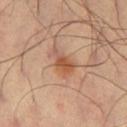  biopsy_status: not biopsied; imaged during a skin examination
  image:
    source: total-body photography crop
    field_of_view_mm: 15
  lighting: cross-polarized
  site: left thigh
  lesion_size:
    long_diameter_mm_approx: 3.5